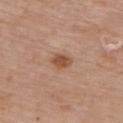Clinical impression: No biopsy was performed on this lesion — it was imaged during a full skin examination and was not determined to be concerning. Image and clinical context: The lesion is located on the back. The subject is a female roughly 65 years of age. A region of skin cropped from a whole-body photographic capture, roughly 15 mm wide. The lesion-visualizer software estimated a mean CIELAB color near L≈51 a*≈21 b*≈32, roughly 11 lightness units darker than nearby skin, and a normalized lesion–skin contrast near 8. And it measured a border-irregularity index near 1.5/10, a within-lesion color-variation index near 2/10, and peripheral color asymmetry of about 0.5.